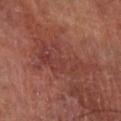Impression:
The lesion was tiled from a total-body skin photograph and was not biopsied.
Clinical summary:
A lesion tile, about 15 mm wide, cut from a 3D total-body photograph. A male subject roughly 70 years of age. Approximately 8.5 mm at its widest. On the leg. The tile uses cross-polarized illumination.